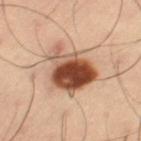The lesion was photographed on a routine skin check and not biopsied; there is no pathology result. Automated image analysis of the tile measured a lesion color around L≈40 a*≈19 b*≈26 in CIELAB, about 17 CIELAB-L* units darker than the surrounding skin, and a normalized lesion–skin contrast near 13. It also reported a border-irregularity index near 4/10, internal color variation of about 9 on a 0–10 scale, and a peripheral color-asymmetry measure near 3. And it measured a detector confidence of about 100 out of 100 that the crop contains a lesion. Cropped from a whole-body photographic skin survey; the tile spans about 15 mm. A male subject, roughly 55 years of age. The lesion is located on the left thigh.